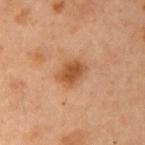biopsy status=total-body-photography surveillance lesion; no biopsy
body site=the left arm
illumination=cross-polarized illumination
acquisition=~15 mm crop, total-body skin-cancer survey
patient=female, aged around 55